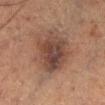Clinical impression:
Captured during whole-body skin photography for melanoma surveillance; the lesion was not biopsied.
Background:
The lesion is located on the left lower leg. A male patient, aged 53 to 57. A roughly 15 mm field-of-view crop from a total-body skin photograph.